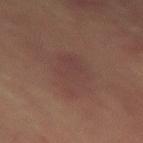Clinical impression:
The lesion was tiled from a total-body skin photograph and was not biopsied.
Image and clinical context:
The lesion-visualizer software estimated a lesion color around L≈35 a*≈17 b*≈19 in CIELAB, about 4 CIELAB-L* units darker than the surrounding skin, and a lesion-to-skin contrast of about 4.5 (normalized; higher = more distinct). And it measured border irregularity of about 3.5 on a 0–10 scale, internal color variation of about 2 on a 0–10 scale, and a peripheral color-asymmetry measure near 1. The tile uses cross-polarized illumination. Measured at roughly 6 mm in maximum diameter. A close-up tile cropped from a whole-body skin photograph, about 15 mm across. A male patient aged around 65. From the back.